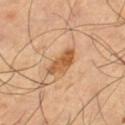biopsy_status: not biopsied; imaged during a skin examination
site: left thigh
lesion_size:
  long_diameter_mm_approx: 4.0
automated_metrics:
  border_irregularity_0_10: 3.0
  color_variation_0_10: 3.5
  peripheral_color_asymmetry: 1.0
  nevus_likeness_0_100: 60
  lesion_detection_confidence_0_100: 100
patient:
  sex: male
  age_approx: 65
image:
  source: total-body photography crop
  field_of_view_mm: 15
lighting: cross-polarized